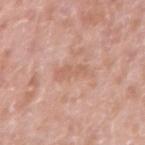Context:
Longest diameter approximately 4 mm. The patient is a male aged 48 to 52. Located on the arm. This image is a 15 mm lesion crop taken from a total-body photograph. The lesion-visualizer software estimated a nevus-likeness score of about 0/100 and a lesion-detection confidence of about 100/100.